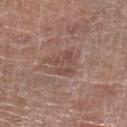Findings:
- notes: total-body-photography surveillance lesion; no biopsy
- tile lighting: white-light
- anatomic site: the leg
- subject: female, in their 80s
- imaging modality: total-body-photography crop, ~15 mm field of view
- size: about 4 mm